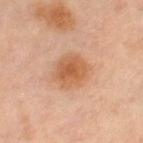notes = imaged on a skin check; not biopsied | image source = ~15 mm tile from a whole-body skin photo | tile lighting = cross-polarized | site = the leg | lesion diameter = ~4 mm (longest diameter) | TBP lesion metrics = a lesion area of about 11 mm², a shape eccentricity near 0.35, and two-axis asymmetry of about 0.2; an average lesion color of about L≈57 a*≈23 b*≈36 (CIELAB), about 10 CIELAB-L* units darker than the surrounding skin, and a normalized border contrast of about 7.5; peripheral color asymmetry of about 1 | patient = female, about 50 years old.